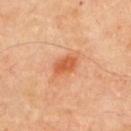Part of a total-body skin-imaging series; this lesion was reviewed on a skin check and was not flagged for biopsy.
Cropped from a total-body skin-imaging series; the visible field is about 15 mm.
The lesion is located on the upper back.
A male patient, in their mid-50s.